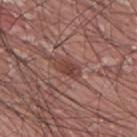<lesion>
  <biopsy_status>not biopsied; imaged during a skin examination</biopsy_status>
  <patient>
    <sex>male</sex>
    <age_approx>40</age_approx>
  </patient>
  <lighting>white-light</lighting>
  <image>
    <source>total-body photography crop</source>
    <field_of_view_mm>15</field_of_view_mm>
  </image>
  <lesion_size>
    <long_diameter_mm_approx>3.0</long_diameter_mm_approx>
  </lesion_size>
  <automated_metrics>
    <cielab_L>42</cielab_L>
    <cielab_a>23</cielab_a>
    <cielab_b>24</cielab_b>
    <vs_skin_darker_L>9.0</vs_skin_darker_L>
    <vs_skin_contrast_norm>7.5</vs_skin_contrast_norm>
    <border_irregularity_0_10>2.5</border_irregularity_0_10>
    <peripheral_color_asymmetry>1.0</peripheral_color_asymmetry>
  </automated_metrics>
  <site>mid back</site>
</lesion>